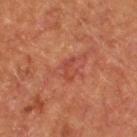follow-up = no biopsy performed (imaged during a skin exam); site = the upper back; illumination = cross-polarized; subject = male, aged approximately 55; acquisition = total-body-photography crop, ~15 mm field of view.